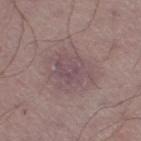Captured during whole-body skin photography for melanoma surveillance; the lesion was not biopsied. This image is a 15 mm lesion crop taken from a total-body photograph. About 4.5 mm across. Imaged with white-light lighting. Located on the leg. A male patient, aged approximately 60.